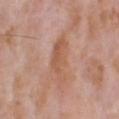workup: imaged on a skin check; not biopsied | illumination: white-light illumination | diameter: ≈5 mm | subject: male, aged approximately 70 | image source: total-body-photography crop, ~15 mm field of view | body site: the head or neck.